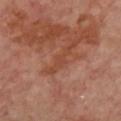| key | value |
|---|---|
| biopsy status | total-body-photography surveillance lesion; no biopsy |
| anatomic site | the chest |
| illumination | cross-polarized |
| acquisition | 15 mm crop, total-body photography |
| patient | in their mid-50s |